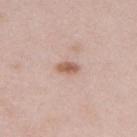Q: Was this lesion biopsied?
A: total-body-photography surveillance lesion; no biopsy
Q: Where on the body is the lesion?
A: the upper back
Q: What are the patient's age and sex?
A: female, approximately 65 years of age
Q: What kind of image is this?
A: total-body-photography crop, ~15 mm field of view